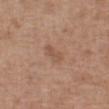Captured during whole-body skin photography for melanoma surveillance; the lesion was not biopsied. A 15 mm crop from a total-body photograph taken for skin-cancer surveillance. A female subject aged 73–77. The recorded lesion diameter is about 3 mm. Automated image analysis of the tile measured a lesion-to-skin contrast of about 5 (normalized; higher = more distinct). It also reported an automated nevus-likeness rating near 0 out of 100 and a lesion-detection confidence of about 100/100. Imaged with white-light lighting. The lesion is on the abdomen.